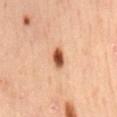Case summary:
– biopsy status · imaged on a skin check; not biopsied
– anatomic site · the back
– patient · male, in their mid- to late 50s
– image source · 15 mm crop, total-body photography